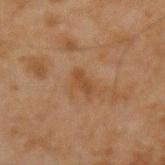Part of a total-body skin-imaging series; this lesion was reviewed on a skin check and was not flagged for biopsy.
A roughly 15 mm field-of-view crop from a total-body skin photograph.
A male subject, in their mid-40s.
The lesion is on the left forearm.
Imaged with cross-polarized lighting.